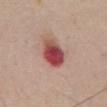workup: total-body-photography surveillance lesion; no biopsy
diameter: ~4 mm (longest diameter)
image source: ~15 mm tile from a whole-body skin photo
tile lighting: white-light
subject: male, aged approximately 55
body site: the abdomen
automated metrics: an area of roughly 10 mm², a shape eccentricity near 0.55, and a symmetry-axis asymmetry near 0.15; a lesion color around L≈48 a*≈30 b*≈23 in CIELAB, about 17 CIELAB-L* units darker than the surrounding skin, and a lesion-to-skin contrast of about 11.5 (normalized; higher = more distinct); radial color variation of about 3.5